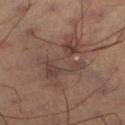Impression: Imaged during a routine full-body skin examination; the lesion was not biopsied and no histopathology is available. Acquisition and patient details: A male subject, roughly 60 years of age. Measured at roughly 6.5 mm in maximum diameter. Automated tile analysis of the lesion measured a lesion area of about 19 mm². It also reported border irregularity of about 8 on a 0–10 scale, a color-variation rating of about 4.5/10, and peripheral color asymmetry of about 1. The software also gave a nevus-likeness score of about 10/100 and a detector confidence of about 80 out of 100 that the crop contains a lesion. Captured under cross-polarized illumination. A 15 mm close-up extracted from a 3D total-body photography capture.